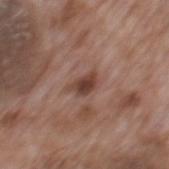Impression:
Recorded during total-body skin imaging; not selected for excision or biopsy.
Background:
The tile uses white-light illumination. The lesion is located on the mid back. The recorded lesion diameter is about 3 mm. A male patient about 70 years old. A region of skin cropped from a whole-body photographic capture, roughly 15 mm wide.